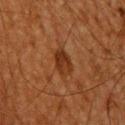A male subject, in their mid- to late 60s. A lesion tile, about 15 mm wide, cut from a 3D total-body photograph. The lesion is located on the upper back. Automated image analysis of the tile measured about 8 CIELAB-L* units darker than the surrounding skin and a lesion-to-skin contrast of about 8 (normalized; higher = more distinct). The analysis additionally found a border-irregularity index near 2/10, a within-lesion color-variation index near 3.5/10, and radial color variation of about 1.5. The analysis additionally found a classifier nevus-likeness of about 35/100 and a detector confidence of about 100 out of 100 that the crop contains a lesion. Imaged with cross-polarized lighting. About 3.5 mm across.